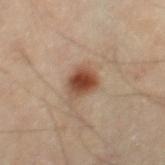Captured during whole-body skin photography for melanoma surveillance; the lesion was not biopsied. On the right thigh. The lesion-visualizer software estimated an area of roughly 7 mm², an outline eccentricity of about 0.55 (0 = round, 1 = elongated), and a shape-asymmetry score of about 0.3 (0 = symmetric). The software also gave an automated nevus-likeness rating near 100 out of 100 and a lesion-detection confidence of about 100/100. Cropped from a whole-body photographic skin survey; the tile spans about 15 mm. The subject is a male roughly 55 years of age.